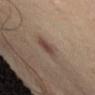Q: Was this lesion biopsied?
A: imaged on a skin check; not biopsied
Q: What is the anatomic site?
A: the abdomen
Q: How was the tile lit?
A: cross-polarized
Q: Lesion size?
A: ~3.5 mm (longest diameter)
Q: Who is the patient?
A: female, aged around 40
Q: What is the imaging modality?
A: ~15 mm crop, total-body skin-cancer survey